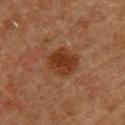| feature | finding |
|---|---|
| workup | catalogued during a skin exam; not biopsied |
| size | ≈4 mm |
| patient | female, roughly 55 years of age |
| image source | ~15 mm crop, total-body skin-cancer survey |
| location | the upper back |
| lighting | cross-polarized |
| TBP lesion metrics | a lesion area of about 10 mm² and an eccentricity of roughly 0.45; a border-irregularity index near 2/10; a classifier nevus-likeness of about 85/100 and a lesion-detection confidence of about 100/100 |